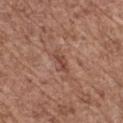The lesion was photographed on a routine skin check and not biopsied; there is no pathology result. The lesion is located on the right thigh. The tile uses white-light illumination. The lesion-visualizer software estimated a lesion area of about 3 mm² and a symmetry-axis asymmetry near 0.4. A female subject, approximately 75 years of age. Approximately 3 mm at its widest. A close-up tile cropped from a whole-body skin photograph, about 15 mm across.